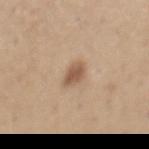The total-body-photography lesion software estimated an eccentricity of roughly 0.7. The software also gave a mean CIELAB color near L≈55 a*≈17 b*≈31, a lesion–skin lightness drop of about 12, and a lesion-to-skin contrast of about 8 (normalized; higher = more distinct). It also reported border irregularity of about 2 on a 0–10 scale, a color-variation rating of about 2/10, and a peripheral color-asymmetry measure near 0.5. The analysis additionally found an automated nevus-likeness rating near 90 out of 100 and a detector confidence of about 100 out of 100 that the crop contains a lesion. Longest diameter approximately 2.5 mm. The lesion is on the chest. The tile uses white-light illumination. A male subject approximately 65 years of age. A roughly 15 mm field-of-view crop from a total-body skin photograph.